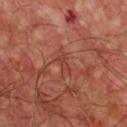<tbp_lesion>
<biopsy_status>not biopsied; imaged during a skin examination</biopsy_status>
<site>front of the torso</site>
<lighting>cross-polarized</lighting>
<patient>
  <sex>male</sex>
  <age_approx>55</age_approx>
</patient>
<image>
  <source>total-body photography crop</source>
  <field_of_view_mm>15</field_of_view_mm>
</image>
</tbp_lesion>